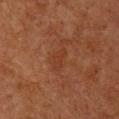<case>
<site>chest</site>
<patient>
  <sex>female</sex>
  <age_approx>40</age_approx>
</patient>
<lighting>cross-polarized</lighting>
<image>
  <source>total-body photography crop</source>
  <field_of_view_mm>15</field_of_view_mm>
</image>
<automated_metrics>
  <cielab_L>32</cielab_L>
  <cielab_a>22</cielab_a>
  <cielab_b>29</cielab_b>
  <vs_skin_contrast_norm>4.5</vs_skin_contrast_norm>
  <nevus_likeness_0_100>0</nevus_likeness_0_100>
</automated_metrics>
<lesion_size>
  <long_diameter_mm_approx>3.0</long_diameter_mm_approx>
</lesion_size>
</case>